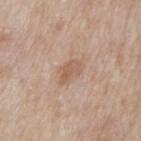biopsy_status: not biopsied; imaged during a skin examination
site: back
patient:
  sex: male
  age_approx: 65
lesion_size:
  long_diameter_mm_approx: 3.5
lighting: white-light
image:
  source: total-body photography crop
  field_of_view_mm: 15
automated_metrics:
  area_mm2_approx: 4.5
  shape_asymmetry: 0.3
  cielab_L: 58
  cielab_a: 18
  cielab_b: 29
  vs_skin_contrast_norm: 6.0
  border_irregularity_0_10: 3.0
  color_variation_0_10: 2.5
  peripheral_color_asymmetry: 1.0